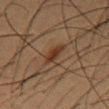{"biopsy_status": "not biopsied; imaged during a skin examination", "lesion_size": {"long_diameter_mm_approx": 4.0}, "image": {"source": "total-body photography crop", "field_of_view_mm": 15}, "lighting": "cross-polarized", "patient": {"sex": "male", "age_approx": 55}, "automated_metrics": {"shape_asymmetry": 0.3, "cielab_L": 30, "cielab_a": 17, "cielab_b": 26, "vs_skin_darker_L": 8.0, "vs_skin_contrast_norm": 8.5, "border_irregularity_0_10": 3.5, "color_variation_0_10": 2.5, "peripheral_color_asymmetry": 1.0, "lesion_detection_confidence_0_100": 100}, "site": "mid back"}